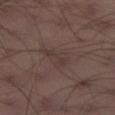Imaged during a routine full-body skin examination; the lesion was not biopsied and no histopathology is available. The lesion is on the leg. The subject is a male roughly 40 years of age. The total-body-photography lesion software estimated a border-irregularity index near 6.5/10 and peripheral color asymmetry of about 0.5. The software also gave an automated nevus-likeness rating near 0 out of 100 and lesion-presence confidence of about 90/100. A region of skin cropped from a whole-body photographic capture, roughly 15 mm wide.